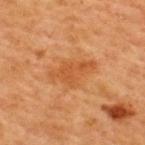Assessment:
No biopsy was performed on this lesion — it was imaged during a full skin examination and was not determined to be concerning.
Acquisition and patient details:
A female subject, in their 40s. Imaged with cross-polarized lighting. The total-body-photography lesion software estimated a border-irregularity rating of about 3.5/10 and a color-variation rating of about 2/10. It also reported a lesion-detection confidence of about 100/100. From the upper back. A 15 mm crop from a total-body photograph taken for skin-cancer surveillance. Measured at roughly 5 mm in maximum diameter.